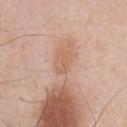Clinical impression:
No biopsy was performed on this lesion — it was imaged during a full skin examination and was not determined to be concerning.
Acquisition and patient details:
On the chest. Captured under white-light illumination. This image is a 15 mm lesion crop taken from a total-body photograph. A male patient approximately 60 years of age. The lesion's longest dimension is about 2.5 mm.